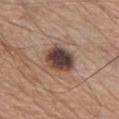Clinical impression:
Captured during whole-body skin photography for melanoma surveillance; the lesion was not biopsied.
Acquisition and patient details:
Captured under white-light illumination. Measured at roughly 4 mm in maximum diameter. A male subject, approximately 70 years of age. A roughly 15 mm field-of-view crop from a total-body skin photograph. Automated tile analysis of the lesion measured an area of roughly 11 mm², a shape eccentricity near 0.6, and a shape-asymmetry score of about 0.2 (0 = symmetric). On the front of the torso.